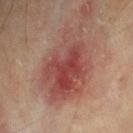{"biopsy_status": "not biopsied; imaged during a skin examination", "image": {"source": "total-body photography crop", "field_of_view_mm": 15}, "patient": {"sex": "male", "age_approx": 75}, "lighting": "cross-polarized", "site": "right upper arm"}